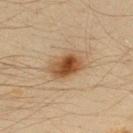Recorded during total-body skin imaging; not selected for excision or biopsy.
A male subject aged 33–37.
A 15 mm close-up tile from a total-body photography series done for melanoma screening.
This is a cross-polarized tile.
The lesion is on the upper back.